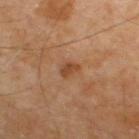From the upper back.
The lesion's longest dimension is about 2.5 mm.
A male subject about 65 years old.
Cropped from a whole-body photographic skin survey; the tile spans about 15 mm.